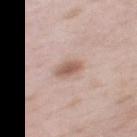The lesion was tiled from a total-body skin photograph and was not biopsied. This is a white-light tile. The subject is a male aged approximately 55. Approximately 3.5 mm at its widest. A lesion tile, about 15 mm wide, cut from a 3D total-body photograph. The lesion-visualizer software estimated a footprint of about 5 mm². The analysis additionally found border irregularity of about 2.5 on a 0–10 scale, a color-variation rating of about 2.5/10, and peripheral color asymmetry of about 0.5. Located on the mid back.